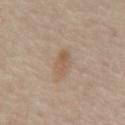Imaged during a routine full-body skin examination; the lesion was not biopsied and no histopathology is available. Cropped from a total-body skin-imaging series; the visible field is about 15 mm. Approximately 3.5 mm at its widest. The lesion-visualizer software estimated a footprint of about 3.5 mm², a shape eccentricity near 0.95, and two-axis asymmetry of about 0.25. It also reported about 8 CIELAB-L* units darker than the surrounding skin. It also reported a border-irregularity index near 3.5/10 and radial color variation of about 0. The software also gave a nevus-likeness score of about 40/100 and a detector confidence of about 100 out of 100 that the crop contains a lesion. On the mid back. A patient roughly 55 years of age. The tile uses cross-polarized illumination.